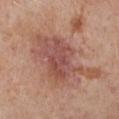biopsy status = no biopsy performed (imaged during a skin exam)
illumination = cross-polarized
size = ≈9 mm
imaging modality = ~15 mm tile from a whole-body skin photo
location = the left arm
patient = female, approximately 40 years of age